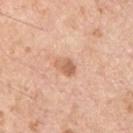follow-up: catalogued during a skin exam; not biopsied | image: 15 mm crop, total-body photography | body site: the chest | automated metrics: a lesion area of about 3.5 mm², an outline eccentricity of about 0.75 (0 = round, 1 = elongated), and two-axis asymmetry of about 0.3; an average lesion color of about L≈63 a*≈23 b*≈34 (CIELAB) and a lesion-to-skin contrast of about 7 (normalized; higher = more distinct) | illumination: white-light illumination | patient: male, about 70 years old.